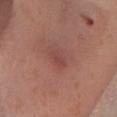Imaged during a routine full-body skin examination; the lesion was not biopsied and no histopathology is available. The lesion is located on the head or neck. Approximately 2.5 mm at its widest. A 15 mm close-up extracted from a 3D total-body photography capture. The lesion-visualizer software estimated border irregularity of about 2.5 on a 0–10 scale, internal color variation of about 1.5 on a 0–10 scale, and peripheral color asymmetry of about 0.5. The patient is a female in their 30s.